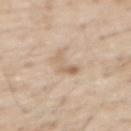biopsy status: no biopsy performed (imaged during a skin exam); patient: male, aged approximately 70; body site: the front of the torso; image source: ~15 mm tile from a whole-body skin photo.